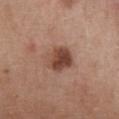Impression:
No biopsy was performed on this lesion — it was imaged during a full skin examination and was not determined to be concerning.
Image and clinical context:
This image is a 15 mm lesion crop taken from a total-body photograph. The patient is a female about 50 years old. This is a white-light tile. The recorded lesion diameter is about 3.5 mm. From the chest. Automated image analysis of the tile measured a lesion area of about 9.5 mm² and an eccentricity of roughly 0.5. It also reported an average lesion color of about L≈45 a*≈22 b*≈27 (CIELAB), about 13 CIELAB-L* units darker than the surrounding skin, and a normalized border contrast of about 9.5. The software also gave a border-irregularity rating of about 1.5/10, a color-variation rating of about 6.5/10, and peripheral color asymmetry of about 2.5. The analysis additionally found a nevus-likeness score of about 85/100.